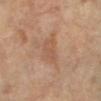Impression: This lesion was catalogued during total-body skin photography and was not selected for biopsy. Context: Automated image analysis of the tile measured a footprint of about 8 mm², a shape eccentricity near 0.8, and a shape-asymmetry score of about 0.3 (0 = symmetric). The analysis additionally found border irregularity of about 3 on a 0–10 scale, a color-variation rating of about 2.5/10, and a peripheral color-asymmetry measure near 1. A female patient roughly 70 years of age. On the left lower leg. Measured at roughly 4 mm in maximum diameter. A roughly 15 mm field-of-view crop from a total-body skin photograph. Imaged with cross-polarized lighting.